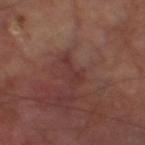Findings:
* workup · imaged on a skin check; not biopsied
* image · 15 mm crop, total-body photography
* anatomic site · the left thigh
* subject · male, aged around 70